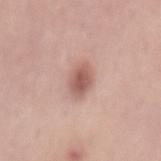follow-up: no biopsy performed (imaged during a skin exam).